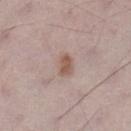patient: male, aged 68 to 72
lighting: white-light illumination
diameter: ~2.5 mm (longest diameter)
site: the left lower leg
imaging modality: ~15 mm crop, total-body skin-cancer survey